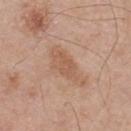Clinical impression:
Part of a total-body skin-imaging series; this lesion was reviewed on a skin check and was not flagged for biopsy.
Acquisition and patient details:
A male patient, roughly 55 years of age. The lesion-visualizer software estimated a lesion area of about 4.5 mm², a shape eccentricity near 0.85, and two-axis asymmetry of about 0.35. It also reported a border-irregularity index near 3.5/10 and a peripheral color-asymmetry measure near 0.5. The software also gave a classifier nevus-likeness of about 0/100 and a detector confidence of about 100 out of 100 that the crop contains a lesion. Imaged with white-light lighting. A 15 mm crop from a total-body photograph taken for skin-cancer surveillance. The lesion is located on the upper back.